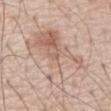Notes:
– automated metrics · a border-irregularity index near 5.5/10, a color-variation rating of about 9/10, and radial color variation of about 3.5; an automated nevus-likeness rating near 0 out of 100 and a detector confidence of about 100 out of 100 that the crop contains a lesion
– image source · ~15 mm crop, total-body skin-cancer survey
– lesion size · ~9 mm (longest diameter)
– anatomic site · the front of the torso
– patient · male, aged approximately 70
– lighting · white-light illumination